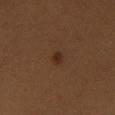This lesion was catalogued during total-body skin photography and was not selected for biopsy.
From the left thigh.
The tile uses cross-polarized illumination.
A 15 mm close-up extracted from a 3D total-body photography capture.
Approximately 2 mm at its widest.
A female patient roughly 40 years of age.
Automated image analysis of the tile measured roughly 7 lightness units darker than nearby skin and a normalized border contrast of about 7.5. The analysis additionally found border irregularity of about 3 on a 0–10 scale, internal color variation of about 0 on a 0–10 scale, and a peripheral color-asymmetry measure near 0.